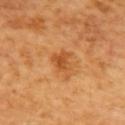biopsy_status: not biopsied; imaged during a skin examination
site: back
patient:
  sex: female
image:
  source: total-body photography crop
  field_of_view_mm: 15